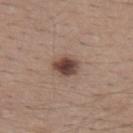Clinical impression:
The lesion was tiled from a total-body skin photograph and was not biopsied.
Background:
The subject is a male aged 38–42. The lesion is on the upper back. The total-body-photography lesion software estimated an eccentricity of roughly 0.6 and a symmetry-axis asymmetry near 0.15. It also reported lesion-presence confidence of about 100/100. The recorded lesion diameter is about 3 mm. A 15 mm crop from a total-body photograph taken for skin-cancer surveillance.